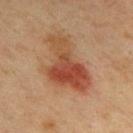Impression: Imaged during a routine full-body skin examination; the lesion was not biopsied and no histopathology is available. Acquisition and patient details: Located on the upper back. The tile uses cross-polarized illumination. Approximately 8 mm at its widest. The patient is a male aged 38 to 42. This image is a 15 mm lesion crop taken from a total-body photograph.